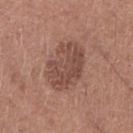Assessment:
No biopsy was performed on this lesion — it was imaged during a full skin examination and was not determined to be concerning.
Context:
Longest diameter approximately 6 mm. Automated tile analysis of the lesion measured a within-lesion color-variation index near 3.5/10. On the leg. A female subject in their 50s. Imaged with white-light lighting. A lesion tile, about 15 mm wide, cut from a 3D total-body photograph.